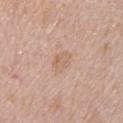Part of a total-body skin-imaging series; this lesion was reviewed on a skin check and was not flagged for biopsy. The tile uses white-light illumination. The lesion is on the arm. This image is a 15 mm lesion crop taken from a total-body photograph. About 2.5 mm across. A male patient aged approximately 70.